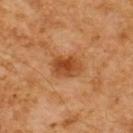Imaged during a routine full-body skin examination; the lesion was not biopsied and no histopathology is available. A roughly 15 mm field-of-view crop from a total-body skin photograph. A male subject, approximately 60 years of age. Approximately 3.5 mm at its widest. From the upper back.